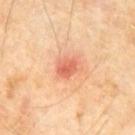The lesion was photographed on a routine skin check and not biopsied; there is no pathology result.